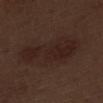{"biopsy_status": "not biopsied; imaged during a skin examination", "image": {"source": "total-body photography crop", "field_of_view_mm": 15}, "site": "left thigh", "patient": {"sex": "male", "age_approx": 70}}